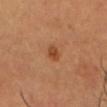Impression: The lesion was tiled from a total-body skin photograph and was not biopsied. Image and clinical context: A male subject in their mid-50s. The lesion is on the head or neck. The total-body-photography lesion software estimated an area of roughly 3 mm² and an eccentricity of roughly 0.6. It also reported roughly 10 lightness units darker than nearby skin and a normalized lesion–skin contrast near 7.5. The software also gave a color-variation rating of about 3.5/10 and radial color variation of about 1.5. It also reported a nevus-likeness score of about 90/100 and a detector confidence of about 100 out of 100 that the crop contains a lesion. A region of skin cropped from a whole-body photographic capture, roughly 15 mm wide. About 2 mm across. This is a cross-polarized tile.